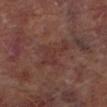Assessment:
The lesion was tiled from a total-body skin photograph and was not biopsied.